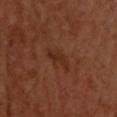{
  "biopsy_status": "not biopsied; imaged during a skin examination",
  "site": "upper back",
  "lighting": "cross-polarized",
  "image": {
    "source": "total-body photography crop",
    "field_of_view_mm": 15
  },
  "patient": {
    "sex": "male",
    "age_approx": 50
  },
  "lesion_size": {
    "long_diameter_mm_approx": 4.0
  }
}